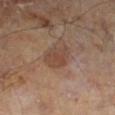Recorded during total-body skin imaging; not selected for excision or biopsy.
The lesion is on the left lower leg.
A 15 mm close-up extracted from a 3D total-body photography capture.
The lesion's longest dimension is about 3.5 mm.
A male subject aged 63 to 67.